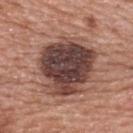Q: Was this lesion biopsied?
A: imaged on a skin check; not biopsied
Q: Where on the body is the lesion?
A: the upper back
Q: How was this image acquired?
A: total-body-photography crop, ~15 mm field of view
Q: What did automated image analysis measure?
A: an area of roughly 34 mm² and a shape-asymmetry score of about 0.2 (0 = symmetric); border irregularity of about 2.5 on a 0–10 scale and a color-variation rating of about 7.5/10; a nevus-likeness score of about 5/100 and a lesion-detection confidence of about 100/100
Q: What is the lesion's diameter?
A: ~7.5 mm (longest diameter)
Q: Patient demographics?
A: female, aged approximately 60
Q: How was the tile lit?
A: white-light